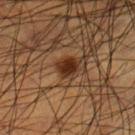Case summary:
– follow-up · total-body-photography surveillance lesion; no biopsy